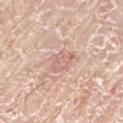This lesion was catalogued during total-body skin photography and was not selected for biopsy.
A 15 mm crop from a total-body photograph taken for skin-cancer surveillance.
The tile uses white-light illumination.
The recorded lesion diameter is about 3.5 mm.
The lesion is on the left upper arm.
A male subject aged approximately 75.